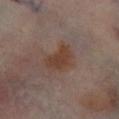This lesion was catalogued during total-body skin photography and was not selected for biopsy. The tile uses cross-polarized illumination. The lesion is on the left lower leg. The recorded lesion diameter is about 4.5 mm. The subject is a male aged 68 to 72. A 15 mm crop from a total-body photograph taken for skin-cancer surveillance.